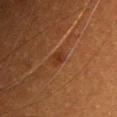Q: Was a biopsy performed?
A: catalogued during a skin exam; not biopsied
Q: Illumination type?
A: cross-polarized
Q: What is the lesion's diameter?
A: ≈1.5 mm
Q: Lesion location?
A: the chest
Q: What kind of image is this?
A: ~15 mm crop, total-body skin-cancer survey
Q: Who is the patient?
A: female, roughly 30 years of age